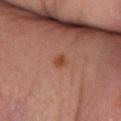Q: What kind of image is this?
A: ~15 mm tile from a whole-body skin photo
Q: Lesion size?
A: ~2 mm (longest diameter)
Q: What did automated image analysis measure?
A: an area of roughly 2.5 mm², an eccentricity of roughly 0.55, and a shape-asymmetry score of about 0.3 (0 = symmetric); a border-irregularity index near 2.5/10, a color-variation rating of about 1/10, and peripheral color asymmetry of about 0.5
Q: What are the patient's age and sex?
A: female, aged approximately 65
Q: How was the tile lit?
A: cross-polarized
Q: What is the anatomic site?
A: the leg